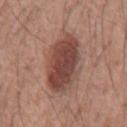Part of a total-body skin-imaging series; this lesion was reviewed on a skin check and was not flagged for biopsy.
A roughly 15 mm field-of-view crop from a total-body skin photograph.
About 6.5 mm across.
A male subject, aged around 55.
Automated image analysis of the tile measured about 13 CIELAB-L* units darker than the surrounding skin and a lesion-to-skin contrast of about 9.5 (normalized; higher = more distinct). It also reported a nevus-likeness score of about 95/100 and a lesion-detection confidence of about 100/100.
Located on the mid back.
This is a white-light tile.